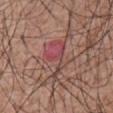Notes:
- biopsy status · imaged on a skin check; not biopsied
- anatomic site · the front of the torso
- imaging modality · 15 mm crop, total-body photography
- lesion diameter · ~5.5 mm (longest diameter)
- patient · male, about 60 years old